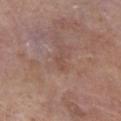Assessment: The lesion was tiled from a total-body skin photograph and was not biopsied. Image and clinical context: Captured under white-light illumination. The lesion is located on the right lower leg. Automated image analysis of the tile measured an area of roughly 2.5 mm². And it measured a lesion–skin lightness drop of about 5 and a normalized lesion–skin contrast near 4.5. A 15 mm close-up extracted from a 3D total-body photography capture. A male patient, aged 78 to 82. Approximately 2.5 mm at its widest.